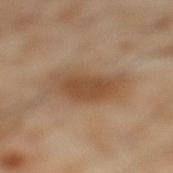patient:
  sex: male
  age_approx: 55
lesion_size:
  long_diameter_mm_approx: 6.0
site: leg
image:
  source: total-body photography crop
  field_of_view_mm: 15